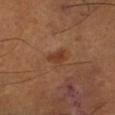Findings:
• notes · catalogued during a skin exam; not biopsied
• site · the right lower leg
• image · total-body-photography crop, ~15 mm field of view
• patient · male, aged 48 to 52
• automated metrics · a symmetry-axis asymmetry near 0.4; a lesion color around L≈37 a*≈23 b*≈31 in CIELAB and a lesion-to-skin contrast of about 7.5 (normalized; higher = more distinct); a border-irregularity rating of about 4/10, internal color variation of about 2 on a 0–10 scale, and radial color variation of about 0.5; a classifier nevus-likeness of about 45/100 and lesion-presence confidence of about 100/100